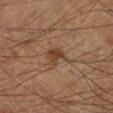Q: Was this lesion biopsied?
A: catalogued during a skin exam; not biopsied
Q: How was this image acquired?
A: ~15 mm crop, total-body skin-cancer survey
Q: What lighting was used for the tile?
A: cross-polarized illumination
Q: What are the patient's age and sex?
A: male, approximately 60 years of age
Q: Automated lesion metrics?
A: a lesion area of about 6 mm² and two-axis asymmetry of about 0.4; a mean CIELAB color near L≈38 a*≈16 b*≈27 and a lesion–skin lightness drop of about 8; a border-irregularity rating of about 4.5/10, internal color variation of about 3.5 on a 0–10 scale, and a peripheral color-asymmetry measure near 1.5
Q: Where on the body is the lesion?
A: the left lower leg